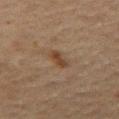  biopsy_status: not biopsied; imaged during a skin examination
  image:
    source: total-body photography crop
    field_of_view_mm: 15
  automated_metrics:
    area_mm2_approx: 3.5
    eccentricity: 0.85
    shape_asymmetry: 0.3
  lesion_size:
    long_diameter_mm_approx: 2.5
  site: abdomen
  patient:
    sex: male
    age_approx: 75
  lighting: cross-polarized